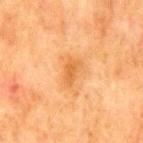No biopsy was performed on this lesion — it was imaged during a full skin examination and was not determined to be concerning.
A male subject, approximately 80 years of age.
The total-body-photography lesion software estimated a lesion area of about 4.5 mm², an eccentricity of roughly 0.85, and a symmetry-axis asymmetry near 0.3. The software also gave about 8 CIELAB-L* units darker than the surrounding skin. And it measured a border-irregularity index near 3/10, a color-variation rating of about 2.5/10, and radial color variation of about 1. The analysis additionally found an automated nevus-likeness rating near 5 out of 100 and lesion-presence confidence of about 100/100.
About 3 mm across.
A close-up tile cropped from a whole-body skin photograph, about 15 mm across.
This is a cross-polarized tile.
Located on the mid back.